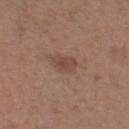notes: no biopsy performed (imaged during a skin exam) | illumination: white-light illumination | imaging modality: ~15 mm tile from a whole-body skin photo | automated lesion analysis: roughly 8 lightness units darker than nearby skin | location: the right thigh | subject: female, aged 28–32.